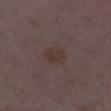Recorded during total-body skin imaging; not selected for excision or biopsy.
A female subject, about 30 years old.
The recorded lesion diameter is about 2.5 mm.
The lesion-visualizer software estimated an automated nevus-likeness rating near 5 out of 100.
A 15 mm crop from a total-body photograph taken for skin-cancer surveillance.
Located on the left lower leg.